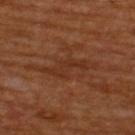Imaged during a routine full-body skin examination; the lesion was not biopsied and no histopathology is available.